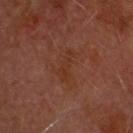Clinical impression: This lesion was catalogued during total-body skin photography and was not selected for biopsy. Background: The subject is a male approximately 60 years of age. The tile uses cross-polarized illumination. The lesion is located on the head or neck. Cropped from a total-body skin-imaging series; the visible field is about 15 mm. Automated tile analysis of the lesion measured a lesion area of about 6.5 mm² and a shape eccentricity near 0.85. And it measured a classifier nevus-likeness of about 0/100 and lesion-presence confidence of about 100/100. The recorded lesion diameter is about 4 mm.